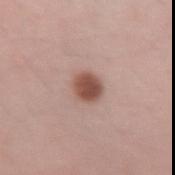Q: What are the patient's age and sex?
A: female, in their mid- to late 30s
Q: How large is the lesion?
A: about 3 mm
Q: Lesion location?
A: the left forearm
Q: How was the tile lit?
A: white-light illumination
Q: What kind of image is this?
A: ~15 mm tile from a whole-body skin photo